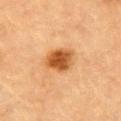<tbp_lesion>
<biopsy_status>not biopsied; imaged during a skin examination</biopsy_status>
<automated_metrics>
  <area_mm2_approx>8.0</area_mm2_approx>
  <eccentricity>0.55</eccentricity>
  <cielab_L>48</cielab_L>
  <cielab_a>24</cielab_a>
  <cielab_b>40</cielab_b>
  <vs_skin_darker_L>14.0</vs_skin_darker_L>
  <vs_skin_contrast_norm>10.5</vs_skin_contrast_norm>
  <nevus_likeness_0_100>100</nevus_likeness_0_100>
  <lesion_detection_confidence_0_100>100</lesion_detection_confidence_0_100>
</automated_metrics>
<lighting>cross-polarized</lighting>
<site>chest</site>
<lesion_size>
  <long_diameter_mm_approx>3.5</long_diameter_mm_approx>
</lesion_size>
<image>
  <source>total-body photography crop</source>
  <field_of_view_mm>15</field_of_view_mm>
</image>
<patient>
  <sex>male</sex>
  <age_approx>85+</age_approx>
</patient>
</tbp_lesion>